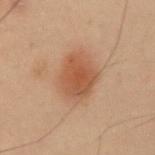This lesion was catalogued during total-body skin photography and was not selected for biopsy.
Longest diameter approximately 5.5 mm.
A region of skin cropped from a whole-body photographic capture, roughly 15 mm wide.
The tile uses cross-polarized illumination.
The subject is a male aged approximately 55.
Automated image analysis of the tile measured a peripheral color-asymmetry measure near 1.
From the right upper arm.